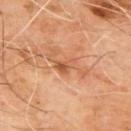Assessment: No biopsy was performed on this lesion — it was imaged during a full skin examination and was not determined to be concerning. Acquisition and patient details: A close-up tile cropped from a whole-body skin photograph, about 15 mm across. Measured at roughly 3 mm in maximum diameter. This is a cross-polarized tile. The lesion-visualizer software estimated a lesion area of about 4 mm² and an eccentricity of roughly 0.75. The software also gave a detector confidence of about 100 out of 100 that the crop contains a lesion. The subject is a male aged 63–67. On the upper back.